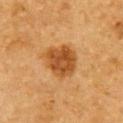<case>
  <biopsy_status>not biopsied; imaged during a skin examination</biopsy_status>
  <image>
    <source>total-body photography crop</source>
    <field_of_view_mm>15</field_of_view_mm>
  </image>
  <site>right upper arm</site>
  <automated_metrics>
    <cielab_L>44</cielab_L>
    <cielab_a>23</cielab_a>
    <cielab_b>39</cielab_b>
    <vs_skin_darker_L>12.0</vs_skin_darker_L>
    <vs_skin_contrast_norm>9.0</vs_skin_contrast_norm>
    <nevus_likeness_0_100>95</nevus_likeness_0_100>
  </automated_metrics>
  <patient>
    <sex>male</sex>
    <age_approx>85+</age_approx>
  </patient>
</case>